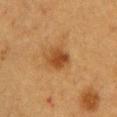Clinical impression:
The lesion was photographed on a routine skin check and not biopsied; there is no pathology result.
Background:
A female patient, approximately 40 years of age. A roughly 15 mm field-of-view crop from a total-body skin photograph. The recorded lesion diameter is about 3 mm. The lesion is located on the chest.